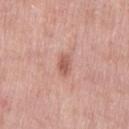Case summary:
* follow-up — imaged on a skin check; not biopsied
* acquisition — total-body-photography crop, ~15 mm field of view
* illumination — white-light
* automated lesion analysis — a footprint of about 4 mm², an outline eccentricity of about 0.85 (0 = round, 1 = elongated), and a shape-asymmetry score of about 0.3 (0 = symmetric); an average lesion color of about L≈58 a*≈25 b*≈27 (CIELAB), about 10 CIELAB-L* units darker than the surrounding skin, and a normalized lesion–skin contrast near 7
* lesion size — ≈3 mm
* location — the left thigh
* subject — female, aged around 40